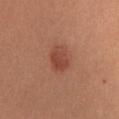Imaged during a routine full-body skin examination; the lesion was not biopsied and no histopathology is available. The patient is a female aged 53–57. The lesion is on the chest. An algorithmic analysis of the crop reported a border-irregularity rating of about 1/10 and peripheral color asymmetry of about 1. Imaged with white-light lighting. A roughly 15 mm field-of-view crop from a total-body skin photograph.